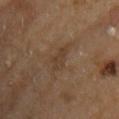Case summary:
- biopsy status: total-body-photography surveillance lesion; no biopsy
- anatomic site: the front of the torso
- size: about 3 mm
- automated lesion analysis: a lesion area of about 4.5 mm², an eccentricity of roughly 0.85, and a shape-asymmetry score of about 0.4 (0 = symmetric); a classifier nevus-likeness of about 0/100 and a detector confidence of about 90 out of 100 that the crop contains a lesion
- image source: ~15 mm tile from a whole-body skin photo
- subject: male, aged 63 to 67